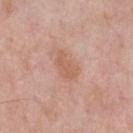Impression: Captured during whole-body skin photography for melanoma surveillance; the lesion was not biopsied. Clinical summary: Longest diameter approximately 3.5 mm. Located on the chest. A male subject, aged around 55. Imaged with white-light lighting. Cropped from a total-body skin-imaging series; the visible field is about 15 mm.